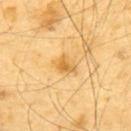The lesion was photographed on a routine skin check and not biopsied; there is no pathology result.
The lesion is on the upper back.
A male subject roughly 65 years of age.
The tile uses cross-polarized illumination.
Cropped from a whole-body photographic skin survey; the tile spans about 15 mm.
About 2.5 mm across.